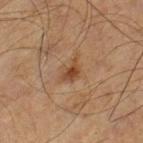Part of a total-body skin-imaging series; this lesion was reviewed on a skin check and was not flagged for biopsy. Automated image analysis of the tile measured about 9 CIELAB-L* units darker than the surrounding skin and a normalized border contrast of about 7.5. About 3 mm across. This is a cross-polarized tile. Cropped from a whole-body photographic skin survey; the tile spans about 15 mm. The patient is a male in their 60s. Located on the left lower leg.